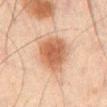• biopsy status — total-body-photography surveillance lesion; no biopsy
• site — the mid back
• image source — 15 mm crop, total-body photography
• subject — male, aged 63–67
• tile lighting — cross-polarized illumination
• lesion size — about 5 mm